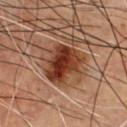Q: What kind of image is this?
A: 15 mm crop, total-body photography
Q: What are the patient's age and sex?
A: male, aged 63–67
Q: What is the lesion's diameter?
A: ~5 mm (longest diameter)
Q: What lighting was used for the tile?
A: cross-polarized
Q: Automated lesion metrics?
A: a lesion area of about 15 mm², a shape eccentricity near 0.65, and a symmetry-axis asymmetry near 0.25; roughly 15 lightness units darker than nearby skin; a nevus-likeness score of about 95/100 and a lesion-detection confidence of about 100/100
Q: What is the anatomic site?
A: the front of the torso